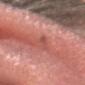Imaged during a routine full-body skin examination; the lesion was not biopsied and no histopathology is available.
A male patient, aged around 30.
Captured under white-light illumination.
On the head or neck.
The lesion-visualizer software estimated a footprint of about 3 mm², a shape eccentricity near 0.9, and a shape-asymmetry score of about 0.4 (0 = symmetric). The analysis additionally found a lesion color around L≈53 a*≈30 b*≈28 in CIELAB and a lesion–skin lightness drop of about 8. The software also gave internal color variation of about 0.5 on a 0–10 scale and radial color variation of about 0.
About 2.5 mm across.
A lesion tile, about 15 mm wide, cut from a 3D total-body photograph.